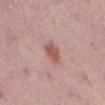Recorded during total-body skin imaging; not selected for excision or biopsy. A female patient, aged around 50. Located on the right lower leg. Automated tile analysis of the lesion measured border irregularity of about 2 on a 0–10 scale and radial color variation of about 0.5. The software also gave a nevus-likeness score of about 75/100. Imaged with white-light lighting. A 15 mm crop from a total-body photograph taken for skin-cancer surveillance.